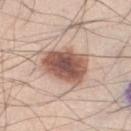subject: male, approximately 35 years of age; imaging modality: ~15 mm tile from a whole-body skin photo; body site: the left lower leg; lighting: white-light illumination; lesion diameter: ≈5 mm; pathology: a dysplastic (Clark) nevus — a benign lesion.